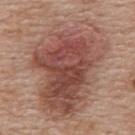Clinical impression:
Captured during whole-body skin photography for melanoma surveillance; the lesion was not biopsied.
Clinical summary:
An algorithmic analysis of the crop reported a border-irregularity rating of about 4/10, internal color variation of about 8 on a 0–10 scale, and a peripheral color-asymmetry measure near 3. The tile uses white-light illumination. A region of skin cropped from a whole-body photographic capture, roughly 15 mm wide. The lesion is on the upper back. About 12 mm across. A female patient, aged 58–62.